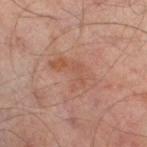Impression: The lesion was tiled from a total-body skin photograph and was not biopsied. Context: A region of skin cropped from a whole-body photographic capture, roughly 15 mm wide. A male subject roughly 65 years of age. On the right thigh.